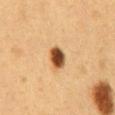Imaged during a routine full-body skin examination; the lesion was not biopsied and no histopathology is available.